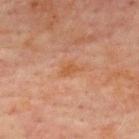The lesion is on the chest.
The patient is a male in their 70s.
A close-up tile cropped from a whole-body skin photograph, about 15 mm across.